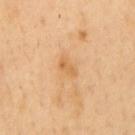| field | value |
|---|---|
| workup | catalogued during a skin exam; not biopsied |
| patient | male, in their 50s |
| diameter | about 2.5 mm |
| illumination | cross-polarized |
| body site | the mid back |
| automated metrics | an average lesion color of about L≈64 a*≈21 b*≈42 (CIELAB), roughly 7 lightness units darker than nearby skin, and a normalized border contrast of about 5.5; a border-irregularity rating of about 3/10, a color-variation rating of about 2/10, and a peripheral color-asymmetry measure near 0.5; an automated nevus-likeness rating near 0 out of 100 |
| image | ~15 mm crop, total-body skin-cancer survey |